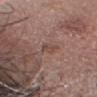Assessment:
Part of a total-body skin-imaging series; this lesion was reviewed on a skin check and was not flagged for biopsy.
Clinical summary:
A male subject aged approximately 55. Cropped from a total-body skin-imaging series; the visible field is about 15 mm. The recorded lesion diameter is about 2.5 mm. Imaged with white-light lighting. Automated image analysis of the tile measured an area of roughly 2.5 mm², an eccentricity of roughly 0.9, and two-axis asymmetry of about 0.3. And it measured a lesion color around L≈46 a*≈19 b*≈24 in CIELAB, roughly 6 lightness units darker than nearby skin, and a normalized border contrast of about 5. The analysis additionally found a border-irregularity index near 3/10. And it measured a lesion-detection confidence of about 100/100. The lesion is on the head or neck.